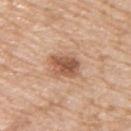biopsy_status: not biopsied; imaged during a skin examination
image:
  source: total-body photography crop
  field_of_view_mm: 15
site: upper back
patient:
  sex: male
  age_approx: 65